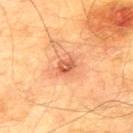Findings:
- notes — no biopsy performed (imaged during a skin exam)
- tile lighting — cross-polarized
- lesion diameter — ≈2.5 mm
- image source — ~15 mm crop, total-body skin-cancer survey
- patient — male, aged around 75
- body site — the upper back